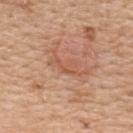{"biopsy_status": "not biopsied; imaged during a skin examination", "image": {"source": "total-body photography crop", "field_of_view_mm": 15}, "site": "upper back", "patient": {"sex": "female", "age_approx": 55}, "lighting": "white-light", "lesion_size": {"long_diameter_mm_approx": 3.0}}